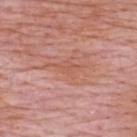Clinical impression: The lesion was photographed on a routine skin check and not biopsied; there is no pathology result. Context: The subject is a male aged 63 to 67. Located on the upper back. A roughly 15 mm field-of-view crop from a total-body skin photograph.